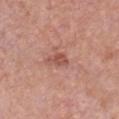The lesion was photographed on a routine skin check and not biopsied; there is no pathology result.
A female subject roughly 40 years of age.
The lesion is on the front of the torso.
Imaged with white-light lighting.
Measured at roughly 2.5 mm in maximum diameter.
A roughly 15 mm field-of-view crop from a total-body skin photograph.